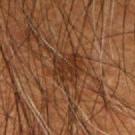Recorded during total-body skin imaging; not selected for excision or biopsy. The subject is a male aged approximately 50. Approximately 3.5 mm at its widest. A roughly 15 mm field-of-view crop from a total-body skin photograph. From the right upper arm.